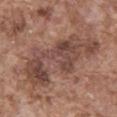<record>
  <biopsy_status>not biopsied; imaged during a skin examination</biopsy_status>
  <image>
    <source>total-body photography crop</source>
    <field_of_view_mm>15</field_of_view_mm>
  </image>
  <site>abdomen</site>
  <patient>
    <sex>male</sex>
    <age_approx>75</age_approx>
  </patient>
</record>